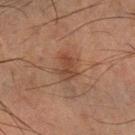Imaged during a routine full-body skin examination; the lesion was not biopsied and no histopathology is available.